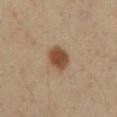notes=imaged on a skin check; not biopsied
acquisition=~15 mm tile from a whole-body skin photo
location=the abdomen
subject=male, aged approximately 60